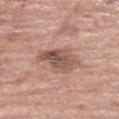follow-up — catalogued during a skin exam; not biopsied
location — the left lower leg
illumination — white-light illumination
image-analysis metrics — a mean CIELAB color near L≈53 a*≈20 b*≈25, a lesion–skin lightness drop of about 12, and a lesion-to-skin contrast of about 8 (normalized; higher = more distinct); lesion-presence confidence of about 100/100
imaging modality — ~15 mm crop, total-body skin-cancer survey
subject — female, roughly 70 years of age